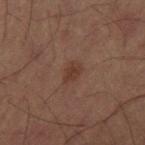This lesion was catalogued during total-body skin photography and was not selected for biopsy. Cropped from a whole-body photographic skin survey; the tile spans about 15 mm. The patient is a male about 60 years old.